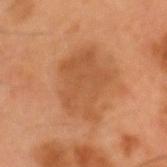Assessment: The lesion was tiled from a total-body skin photograph and was not biopsied. Acquisition and patient details: Captured under cross-polarized illumination. Located on the head or neck. A male subject, roughly 55 years of age. The total-body-photography lesion software estimated a footprint of about 22 mm², an eccentricity of roughly 0.7, and two-axis asymmetry of about 0.35. It also reported a lesion color around L≈48 a*≈23 b*≈36 in CIELAB, a lesion–skin lightness drop of about 7, and a normalized border contrast of about 5.5. The analysis additionally found a border-irregularity index near 4.5/10, a within-lesion color-variation index near 2.5/10, and peripheral color asymmetry of about 1. The analysis additionally found a classifier nevus-likeness of about 45/100 and lesion-presence confidence of about 100/100. A roughly 15 mm field-of-view crop from a total-body skin photograph.